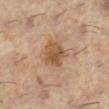A roughly 15 mm field-of-view crop from a total-body skin photograph.
Located on the left lower leg.
The lesion's longest dimension is about 3.5 mm.
The tile uses cross-polarized illumination.
Automated image analysis of the tile measured a lesion area of about 8 mm², a shape eccentricity near 0.55, and a symmetry-axis asymmetry near 0.2.
A female subject, about 60 years old.